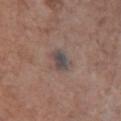The lesion was photographed on a routine skin check and not biopsied; there is no pathology result.
The tile uses white-light illumination.
Measured at roughly 3 mm in maximum diameter.
The lesion-visualizer software estimated an outline eccentricity of about 0.65 (0 = round, 1 = elongated) and a symmetry-axis asymmetry near 0.2. The software also gave a nevus-likeness score of about 0/100 and a detector confidence of about 100 out of 100 that the crop contains a lesion.
On the chest.
A female patient in their mid-60s.
A roughly 15 mm field-of-view crop from a total-body skin photograph.